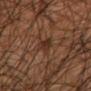biopsy status: no biopsy performed (imaged during a skin exam) | lesion diameter: ≈3.5 mm | automated lesion analysis: a footprint of about 4 mm² and a shape-asymmetry score of about 0.3 (0 = symmetric); a mean CIELAB color near L≈29 a*≈17 b*≈24, roughly 7 lightness units darker than nearby skin, and a lesion-to-skin contrast of about 7.5 (normalized; higher = more distinct); border irregularity of about 3 on a 0–10 scale, a within-lesion color-variation index near 1.5/10, and radial color variation of about 0.5 | subject: male, approximately 45 years of age | image source: ~15 mm tile from a whole-body skin photo | body site: the right upper arm | illumination: cross-polarized.